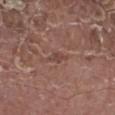| feature | finding |
|---|---|
| follow-up | total-body-photography surveillance lesion; no biopsy |
| image source | total-body-photography crop, ~15 mm field of view |
| subject | male, aged around 70 |
| body site | the right lower leg |